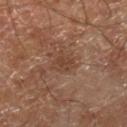{"biopsy_status": "not biopsied; imaged during a skin examination", "lesion_size": {"long_diameter_mm_approx": 3.0}, "lighting": "cross-polarized", "automated_metrics": {"cielab_L": 40, "cielab_a": 19, "cielab_b": 28, "vs_skin_darker_L": 7.0, "vs_skin_contrast_norm": 5.5}, "patient": {"sex": "male", "age_approx": 70}, "site": "right lower leg", "image": {"source": "total-body photography crop", "field_of_view_mm": 15}}